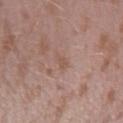{
  "biopsy_status": "not biopsied; imaged during a skin examination",
  "image": {
    "source": "total-body photography crop",
    "field_of_view_mm": 15
  },
  "site": "left forearm",
  "lighting": "white-light",
  "automated_metrics": {
    "cielab_L": 54,
    "cielab_a": 19,
    "cielab_b": 25,
    "vs_skin_contrast_norm": 4.5,
    "border_irregularity_0_10": 3.0,
    "color_variation_0_10": 0.0,
    "peripheral_color_asymmetry": 0.0,
    "nevus_likeness_0_100": 0,
    "lesion_detection_confidence_0_100": 100
  },
  "patient": {
    "sex": "male",
    "age_approx": 40
  }
}